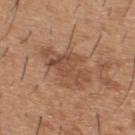Located on the upper back. A lesion tile, about 15 mm wide, cut from a 3D total-body photograph. A male patient, aged 38 to 42. Captured under white-light illumination. The lesion's longest dimension is about 6 mm.